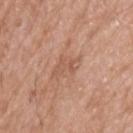Captured during whole-body skin photography for melanoma surveillance; the lesion was not biopsied. From the upper back. This is a white-light tile. Automated tile analysis of the lesion measured a lesion area of about 5.5 mm², a shape eccentricity near 0.75, and two-axis asymmetry of about 0.4. And it measured border irregularity of about 4.5 on a 0–10 scale and a within-lesion color-variation index near 2/10. About 3.5 mm across. This image is a 15 mm lesion crop taken from a total-body photograph. A male patient roughly 65 years of age.